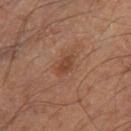Captured during whole-body skin photography for melanoma surveillance; the lesion was not biopsied.
Cropped from a whole-body photographic skin survey; the tile spans about 15 mm.
Located on the right thigh.
A male patient approximately 55 years of age.